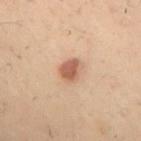This lesion was catalogued during total-body skin photography and was not selected for biopsy.
On the upper back.
Imaged with cross-polarized lighting.
The patient is a male in their 50s.
A region of skin cropped from a whole-body photographic capture, roughly 15 mm wide.
Measured at roughly 3 mm in maximum diameter.